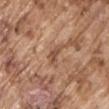Notes:
• workup: catalogued during a skin exam; not biopsied
• subject: male, aged 73 to 77
• lesion size: ~3 mm (longest diameter)
• lighting: white-light illumination
• acquisition: ~15 mm tile from a whole-body skin photo
• site: the right upper arm